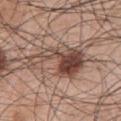No biopsy was performed on this lesion — it was imaged during a full skin examination and was not determined to be concerning. A 15 mm close-up extracted from a 3D total-body photography capture. The lesion is on the chest. A male patient aged 68–72.